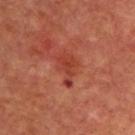Assessment: The lesion was photographed on a routine skin check and not biopsied; there is no pathology result.